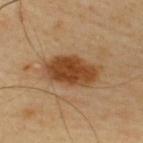notes: catalogued during a skin exam; not biopsied | size: ~6 mm (longest diameter) | site: the upper back | tile lighting: cross-polarized illumination | acquisition: total-body-photography crop, ~15 mm field of view | patient: male, roughly 65 years of age.